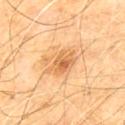Findings:
– biopsy status: total-body-photography surveillance lesion; no biopsy
– size: ≈4 mm
– acquisition: ~15 mm tile from a whole-body skin photo
– automated metrics: a footprint of about 8 mm² and a shape-asymmetry score of about 0.3 (0 = symmetric); a lesion color around L≈64 a*≈23 b*≈44 in CIELAB and roughly 11 lightness units darker than nearby skin
– site: the front of the torso
– patient: male, aged 58–62